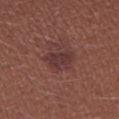Assessment: Recorded during total-body skin imaging; not selected for excision or biopsy. Background: The lesion is on the right upper arm. Cropped from a whole-body photographic skin survey; the tile spans about 15 mm. Measured at roughly 4 mm in maximum diameter. The patient is a female approximately 25 years of age.